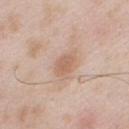| field | value |
|---|---|
| workup | catalogued during a skin exam; not biopsied |
| patient | male, roughly 55 years of age |
| anatomic site | the front of the torso |
| illumination | white-light illumination |
| acquisition | 15 mm crop, total-body photography |